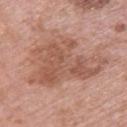Case summary:
– follow-up — no biopsy performed (imaged during a skin exam)
– image — ~15 mm tile from a whole-body skin photo
– patient — female, roughly 60 years of age
– lighting — white-light illumination
– anatomic site — the upper back